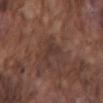Clinical impression:
Imaged during a routine full-body skin examination; the lesion was not biopsied and no histopathology is available.
Context:
Automated image analysis of the tile measured an area of roughly 4 mm² and a shape-asymmetry score of about 0.55 (0 = symmetric). It also reported about 5 CIELAB-L* units darker than the surrounding skin and a normalized border contrast of about 5.5. The analysis additionally found internal color variation of about 1.5 on a 0–10 scale and a peripheral color-asymmetry measure near 0.5. Cropped from a total-body skin-imaging series; the visible field is about 15 mm. A male subject in their mid- to late 70s. From the back. This is a white-light tile. About 3 mm across.